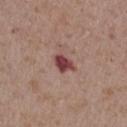The lesion was photographed on a routine skin check and not biopsied; there is no pathology result. A male subject, about 70 years old. An algorithmic analysis of the crop reported an average lesion color of about L≈43 a*≈26 b*≈20 (CIELAB), roughly 15 lightness units darker than nearby skin, and a normalized border contrast of about 11. It also reported a color-variation rating of about 3/10. It also reported a nevus-likeness score of about 5/100 and lesion-presence confidence of about 100/100. Longest diameter approximately 3 mm. The tile uses white-light illumination. A 15 mm close-up extracted from a 3D total-body photography capture. Located on the left upper arm.